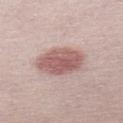Findings:
– biopsy status — no biopsy performed (imaged during a skin exam)
– lesion diameter — about 5.5 mm
– image — ~15 mm tile from a whole-body skin photo
– automated metrics — a footprint of about 16 mm², a shape eccentricity near 0.75, and a symmetry-axis asymmetry near 0.1; roughly 13 lightness units darker than nearby skin and a normalized lesion–skin contrast near 8.5
– subject — male, roughly 30 years of age
– illumination — white-light